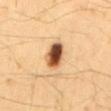Q: What lighting was used for the tile?
A: cross-polarized illumination
Q: What kind of image is this?
A: 15 mm crop, total-body photography
Q: What did automated image analysis measure?
A: a color-variation rating of about 10/10 and a peripheral color-asymmetry measure near 3.5; an automated nevus-likeness rating near 100 out of 100 and a detector confidence of about 100 out of 100 that the crop contains a lesion
Q: What are the patient's age and sex?
A: male, aged 63 to 67
Q: What is the anatomic site?
A: the mid back